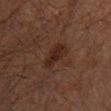About 4 mm across.
A male subject aged 58–62.
On the mid back.
A 15 mm close-up extracted from a 3D total-body photography capture.
Automated image analysis of the tile measured a footprint of about 6 mm², an eccentricity of roughly 0.9, and a symmetry-axis asymmetry near 0.25. It also reported a border-irregularity rating of about 3/10 and internal color variation of about 2.5 on a 0–10 scale.